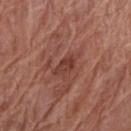Impression:
Imaged during a routine full-body skin examination; the lesion was not biopsied and no histopathology is available.
Image and clinical context:
Measured at roughly 3 mm in maximum diameter. A female patient, in their 80s. Located on the right forearm. Captured under white-light illumination. A close-up tile cropped from a whole-body skin photograph, about 15 mm across.